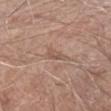biopsy status: imaged on a skin check; not biopsied
subject: male, about 80 years old
location: the right forearm
diameter: ≈3 mm
tile lighting: white-light
acquisition: 15 mm crop, total-body photography